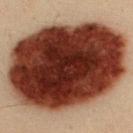Background:
From the upper back. A lesion tile, about 15 mm wide, cut from a 3D total-body photograph. A male patient in their mid- to late 30s. Automated image analysis of the tile measured two-axis asymmetry of about 0.05. The software also gave a mean CIELAB color near L≈31 a*≈23 b*≈26 and about 28 CIELAB-L* units darker than the surrounding skin. The software also gave a border-irregularity index near 1/10, internal color variation of about 9.5 on a 0–10 scale, and radial color variation of about 3.